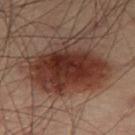<record>
<patient>
  <sex>male</sex>
  <age_approx>55</age_approx>
</patient>
<image>
  <source>total-body photography crop</source>
  <field_of_view_mm>15</field_of_view_mm>
</image>
<lighting>cross-polarized</lighting>
<lesion_size>
  <long_diameter_mm_approx>8.5</long_diameter_mm_approx>
</lesion_size>
<site>leg</site>
</record>